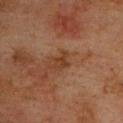Clinical impression:
Recorded during total-body skin imaging; not selected for excision or biopsy.
Background:
A roughly 15 mm field-of-view crop from a total-body skin photograph. The lesion's longest dimension is about 2.5 mm. A male subject in their mid- to late 70s. On the upper back. Captured under cross-polarized illumination.